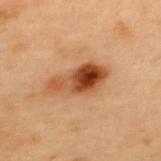  biopsy_status: not biopsied; imaged during a skin examination
  lighting: cross-polarized
  patient:
    sex: male
    age_approx: 55
  automated_metrics:
    cielab_L: 39
    cielab_a: 22
    cielab_b: 32
    vs_skin_darker_L: 14.0
  site: upper back
  lesion_size:
    long_diameter_mm_approx: 6.0
  image:
    source: total-body photography crop
    field_of_view_mm: 15